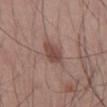Q: Is there a histopathology result?
A: imaged on a skin check; not biopsied
Q: Illumination type?
A: white-light
Q: What kind of image is this?
A: 15 mm crop, total-body photography
Q: What is the anatomic site?
A: the right thigh
Q: Patient demographics?
A: male, about 55 years old